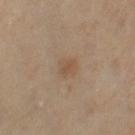Assessment: Captured during whole-body skin photography for melanoma surveillance; the lesion was not biopsied. Acquisition and patient details: On the right thigh. The subject is a female roughly 50 years of age. A 15 mm close-up tile from a total-body photography series done for melanoma screening.